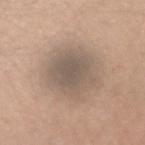Longest diameter approximately 5.5 mm.
Imaged with white-light lighting.
A female patient, aged 18 to 22.
A 15 mm crop from a total-body photograph taken for skin-cancer surveillance.
From the right forearm.